The tile uses white-light illumination. The lesion is on the left thigh. The patient is a female roughly 30 years of age. The total-body-photography lesion software estimated roughly 17 lightness units darker than nearby skin and a normalized lesion–skin contrast near 11.5. The software also gave a border-irregularity rating of about 1.5/10 and peripheral color asymmetry of about 1.5. The analysis additionally found a nevus-likeness score of about 95/100 and a lesion-detection confidence of about 100/100. A 15 mm crop from a total-body photograph taken for skin-cancer surveillance. Longest diameter approximately 3 mm.
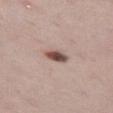diagnosis = a dysplastic (Clark) nevus.